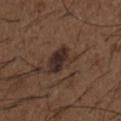Findings:
• acquisition: 15 mm crop, total-body photography
• subject: male, aged approximately 50
• site: the chest